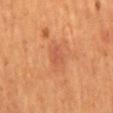* site: the mid back
* lesion size: ≈2.5 mm
* patient: male, aged 53 to 57
* image source: 15 mm crop, total-body photography